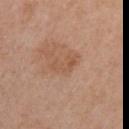Captured under white-light illumination. The patient is a female about 55 years old. A 15 mm crop from a total-body photograph taken for skin-cancer surveillance. The lesion is located on the right upper arm. Measured at roughly 3.5 mm in maximum diameter.